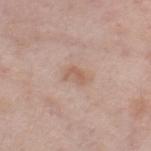Imaged during a routine full-body skin examination; the lesion was not biopsied and no histopathology is available. The patient is a female approximately 65 years of age. About 2.5 mm across. The lesion-visualizer software estimated an average lesion color of about L≈59 a*≈18 b*≈28 (CIELAB) and about 7 CIELAB-L* units darker than the surrounding skin. The tile uses white-light illumination. A 15 mm close-up extracted from a 3D total-body photography capture. The lesion is on the right thigh.